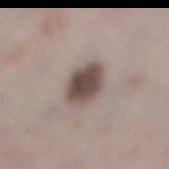The lesion was tiled from a total-body skin photograph and was not biopsied. Approximately 4 mm at its widest. Located on the right lower leg. The subject is a female aged approximately 50. The lesion-visualizer software estimated an area of roughly 11 mm², an outline eccentricity of about 0.5 (0 = round, 1 = elongated), and a shape-asymmetry score of about 0.15 (0 = symmetric). And it measured a mean CIELAB color near L≈48 a*≈13 b*≈18, about 15 CIELAB-L* units darker than the surrounding skin, and a lesion-to-skin contrast of about 11 (normalized; higher = more distinct). The software also gave a nevus-likeness score of about 60/100 and lesion-presence confidence of about 100/100. A region of skin cropped from a whole-body photographic capture, roughly 15 mm wide.